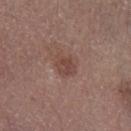Impression: The lesion was photographed on a routine skin check and not biopsied; there is no pathology result. Context: From the left lower leg. Cropped from a total-body skin-imaging series; the visible field is about 15 mm. The patient is a male roughly 75 years of age.